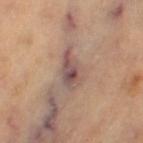  biopsy_status: not biopsied; imaged during a skin examination
  automated_metrics:
    vs_skin_darker_L: 11.0
    vs_skin_contrast_norm: 8.5
    nevus_likeness_0_100: 0
    lesion_detection_confidence_0_100: 55
  lesion_size:
    long_diameter_mm_approx: 5.5
  lighting: cross-polarized
  image:
    source: total-body photography crop
    field_of_view_mm: 15
  site: left thigh
  patient:
    sex: female
    age_approx: 70